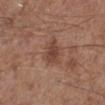* anatomic site: the left lower leg
* subject: male, roughly 60 years of age
* image source: ~15 mm tile from a whole-body skin photo